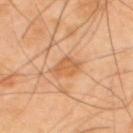Clinical impression:
Recorded during total-body skin imaging; not selected for excision or biopsy.
Acquisition and patient details:
Located on the upper back. A 15 mm close-up extracted from a 3D total-body photography capture. About 2.5 mm across. Automated tile analysis of the lesion measured an average lesion color of about L≈61 a*≈25 b*≈41 (CIELAB), roughly 9 lightness units darker than nearby skin, and a lesion-to-skin contrast of about 6.5 (normalized; higher = more distinct). The analysis additionally found a classifier nevus-likeness of about 0/100 and a detector confidence of about 100 out of 100 that the crop contains a lesion. A male subject aged 38 to 42.